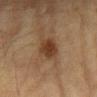Q: Was a biopsy performed?
A: imaged on a skin check; not biopsied
Q: Patient demographics?
A: male, about 85 years old or older
Q: How was this image acquired?
A: ~15 mm crop, total-body skin-cancer survey
Q: What is the lesion's diameter?
A: ~3.5 mm (longest diameter)
Q: Where on the body is the lesion?
A: the abdomen
Q: Illumination type?
A: cross-polarized
Q: Automated lesion metrics?
A: an average lesion color of about L≈33 a*≈17 b*≈28 (CIELAB) and a lesion–skin lightness drop of about 9; border irregularity of about 2 on a 0–10 scale and a within-lesion color-variation index near 3/10